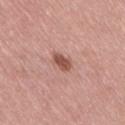Part of a total-body skin-imaging series; this lesion was reviewed on a skin check and was not flagged for biopsy.
The lesion is on the right thigh.
This is a white-light tile.
Automated tile analysis of the lesion measured a border-irregularity index near 2.5/10 and peripheral color asymmetry of about 0.5.
A female subject aged around 40.
A 15 mm close-up tile from a total-body photography series done for melanoma screening.
Longest diameter approximately 2.5 mm.